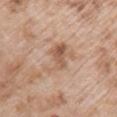<case>
<lighting>white-light</lighting>
<lesion_size>
  <long_diameter_mm_approx>5.5</long_diameter_mm_approx>
</lesion_size>
<patient>
  <sex>female</sex>
  <age_approx>70</age_approx>
</patient>
<automated_metrics>
  <area_mm2_approx>12.0</area_mm2_approx>
  <eccentricity>0.85</eccentricity>
  <shape_asymmetry>0.35</shape_asymmetry>
</automated_metrics>
<image>
  <source>total-body photography crop</source>
  <field_of_view_mm>15</field_of_view_mm>
</image>
<site>left upper arm</site>
</case>